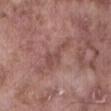Acquisition and patient details: Longest diameter approximately 4.5 mm. Cropped from a total-body skin-imaging series; the visible field is about 15 mm. A male patient aged around 75. Located on the abdomen. An algorithmic analysis of the crop reported an area of roughly 5 mm² and an eccentricity of roughly 0.95. The software also gave a normalized lesion–skin contrast near 5.5. The software also gave a border-irregularity rating of about 8/10, a color-variation rating of about 1/10, and radial color variation of about 0.5.